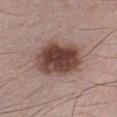Clinical impression: No biopsy was performed on this lesion — it was imaged during a full skin examination and was not determined to be concerning. Image and clinical context: An algorithmic analysis of the crop reported a lesion area of about 25 mm², a shape eccentricity near 0.7, and two-axis asymmetry of about 0.15. It also reported an average lesion color of about L≈44 a*≈19 b*≈23 (CIELAB), about 16 CIELAB-L* units darker than the surrounding skin, and a normalized border contrast of about 11.5. And it measured a classifier nevus-likeness of about 90/100. This is a white-light tile. Cropped from a total-body skin-imaging series; the visible field is about 15 mm. On the chest. A male subject, aged around 25. Approximately 6.5 mm at its widest.